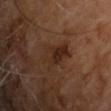follow-up — total-body-photography surveillance lesion; no biopsy
imaging modality — 15 mm crop, total-body photography
automated metrics — an eccentricity of roughly 0.85 and a shape-asymmetry score of about 0.3 (0 = symmetric); a border-irregularity rating of about 4.5/10 and a within-lesion color-variation index near 4/10; a classifier nevus-likeness of about 5/100 and lesion-presence confidence of about 100/100
lighting — cross-polarized
diameter — about 4.5 mm
anatomic site — the upper back
patient — male, aged 58 to 62